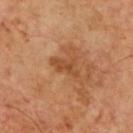notes: no biopsy performed (imaged during a skin exam) | image: 15 mm crop, total-body photography | lighting: cross-polarized illumination | lesion size: ~3.5 mm (longest diameter) | subject: male, about 65 years old | automated metrics: an area of roughly 5 mm² and an eccentricity of roughly 0.85; an average lesion color of about L≈47 a*≈24 b*≈37 (CIELAB), about 8 CIELAB-L* units darker than the surrounding skin, and a normalized lesion–skin contrast near 6.5.